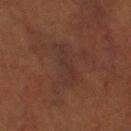The lesion was photographed on a routine skin check and not biopsied; there is no pathology result. The total-body-photography lesion software estimated a footprint of about 9 mm² and two-axis asymmetry of about 0.4. The software also gave a lesion color around L≈23 a*≈14 b*≈18 in CIELAB and roughly 3 lightness units darker than nearby skin. It also reported a color-variation rating of about 2.5/10 and radial color variation of about 1. Captured under cross-polarized illumination. Cropped from a whole-body photographic skin survey; the tile spans about 15 mm. On the right lower leg. About 5.5 mm across. The patient is a female aged 78–82.